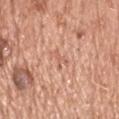{"biopsy_status": "not biopsied; imaged during a skin examination", "patient": {"sex": "male", "age_approx": 70}, "image": {"source": "total-body photography crop", "field_of_view_mm": 15}, "site": "head or neck"}